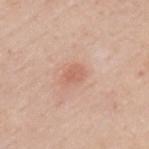notes: catalogued during a skin exam; not biopsied
location: the left upper arm
automated metrics: a footprint of about 3.5 mm² and a shape-asymmetry score of about 0.25 (0 = symmetric); border irregularity of about 2.5 on a 0–10 scale and radial color variation of about 0.5
lighting: white-light illumination
imaging modality: 15 mm crop, total-body photography
patient: male, approximately 50 years of age